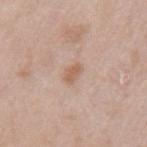{
  "lesion_size": {
    "long_diameter_mm_approx": 2.5
  },
  "patient": {
    "sex": "female",
    "age_approx": 40
  },
  "lighting": "white-light",
  "site": "left upper arm",
  "automated_metrics": {
    "area_mm2_approx": 3.5,
    "border_irregularity_0_10": 2.5,
    "color_variation_0_10": 1.0,
    "peripheral_color_asymmetry": 0.5,
    "nevus_likeness_0_100": 40,
    "lesion_detection_confidence_0_100": 100
  },
  "image": {
    "source": "total-body photography crop",
    "field_of_view_mm": 15
  }
}